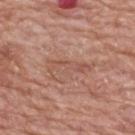workup: total-body-photography surveillance lesion; no biopsy | body site: the upper back | image: ~15 mm crop, total-body skin-cancer survey | lesion diameter: ~5.5 mm (longest diameter) | subject: female, roughly 60 years of age.